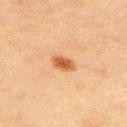Part of a total-body skin-imaging series; this lesion was reviewed on a skin check and was not flagged for biopsy.
On the front of the torso.
Automated tile analysis of the lesion measured a footprint of about 4.5 mm², an outline eccentricity of about 0.8 (0 = round, 1 = elongated), and a symmetry-axis asymmetry near 0.2. And it measured about 11 CIELAB-L* units darker than the surrounding skin. It also reported a border-irregularity index near 1.5/10.
The subject is a male about 65 years old.
This image is a 15 mm lesion crop taken from a total-body photograph.